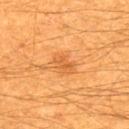<record>
<biopsy_status>not biopsied; imaged during a skin examination</biopsy_status>
<lighting>cross-polarized</lighting>
<site>upper back</site>
<patient>
  <sex>male</sex>
  <age_approx>60</age_approx>
</patient>
<image>
  <source>total-body photography crop</source>
  <field_of_view_mm>15</field_of_view_mm>
</image>
<lesion_size>
  <long_diameter_mm_approx>3.0</long_diameter_mm_approx>
</lesion_size>
</record>